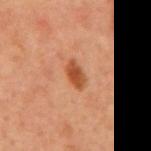Impression: Part of a total-body skin-imaging series; this lesion was reviewed on a skin check and was not flagged for biopsy. Clinical summary: A region of skin cropped from a whole-body photographic capture, roughly 15 mm wide. The lesion is on the chest. Automated image analysis of the tile measured a footprint of about 4.5 mm² and a symmetry-axis asymmetry near 0.25. It also reported roughly 11 lightness units darker than nearby skin and a lesion-to-skin contrast of about 9 (normalized; higher = more distinct). It also reported an automated nevus-likeness rating near 90 out of 100 and a lesion-detection confidence of about 100/100. A male subject approximately 60 years of age.